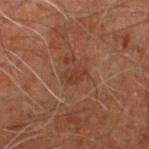Impression:
Part of a total-body skin-imaging series; this lesion was reviewed on a skin check and was not flagged for biopsy.
Context:
On the right leg. The tile uses cross-polarized illumination. This image is a 15 mm lesion crop taken from a total-body photograph. Automated tile analysis of the lesion measured a border-irregularity rating of about 2.5/10 and radial color variation of about 1.5. A male subject about 60 years old.